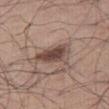Q: Is there a histopathology result?
A: imaged on a skin check; not biopsied
Q: Patient demographics?
A: male, in their mid- to late 60s
Q: What is the imaging modality?
A: 15 mm crop, total-body photography
Q: Lesion location?
A: the right thigh